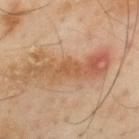Recorded during total-body skin imaging; not selected for excision or biopsy.
The subject is a male about 55 years old.
The lesion is on the mid back.
A 15 mm crop from a total-body photograph taken for skin-cancer surveillance.